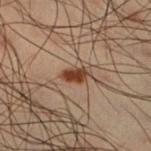The lesion was tiled from a total-body skin photograph and was not biopsied.